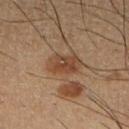Captured during whole-body skin photography for melanoma surveillance; the lesion was not biopsied. A roughly 15 mm field-of-view crop from a total-body skin photograph. A male patient, aged approximately 40. The lesion-visualizer software estimated a footprint of about 9 mm² and a shape eccentricity near 0.7. The analysis additionally found an average lesion color of about L≈44 a*≈19 b*≈30 (CIELAB), roughly 9 lightness units darker than nearby skin, and a normalized lesion–skin contrast near 7.5. And it measured a border-irregularity index near 2/10, a color-variation rating of about 4/10, and a peripheral color-asymmetry measure near 1.5. The software also gave a nevus-likeness score of about 100/100 and lesion-presence confidence of about 100/100. From the right lower leg. Measured at roughly 4 mm in maximum diameter. Captured under cross-polarized illumination.